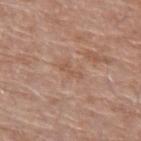Assessment: Part of a total-body skin-imaging series; this lesion was reviewed on a skin check and was not flagged for biopsy. Background: A region of skin cropped from a whole-body photographic capture, roughly 15 mm wide. The lesion is on the right upper arm. The patient is a male roughly 70 years of age. The tile uses white-light illumination.